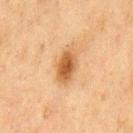workup: no biopsy performed (imaged during a skin exam)
lighting: cross-polarized
imaging modality: total-body-photography crop, ~15 mm field of view
image-analysis metrics: a lesion area of about 7 mm², an outline eccentricity of about 0.8 (0 = round, 1 = elongated), and a symmetry-axis asymmetry near 0.15; a border-irregularity rating of about 1.5/10 and a peripheral color-asymmetry measure near 1
lesion diameter: about 4 mm
body site: the chest
patient: male, approximately 75 years of age